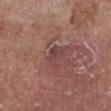Captured during whole-body skin photography for melanoma surveillance; the lesion was not biopsied. The subject is a female in their 80s. A region of skin cropped from a whole-body photographic capture, roughly 15 mm wide. The lesion is located on the front of the torso.